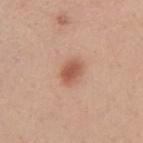<tbp_lesion>
<biopsy_status>not biopsied; imaged during a skin examination</biopsy_status>
<site>chest</site>
<patient>
  <sex>female</sex>
  <age_approx>30</age_approx>
</patient>
<image>
  <source>total-body photography crop</source>
  <field_of_view_mm>15</field_of_view_mm>
</image>
</tbp_lesion>